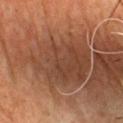follow-up: imaged on a skin check; not biopsied
site: the chest
imaging modality: ~15 mm crop, total-body skin-cancer survey
patient: male, roughly 60 years of age